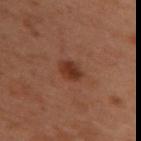Acquisition and patient details:
This image is a 15 mm lesion crop taken from a total-body photograph. A female subject approximately 55 years of age. Located on the upper back. About 3 mm across. Captured under cross-polarized illumination.